About 3.5 mm across, a 15 mm close-up extracted from a 3D total-body photography capture, the subject is a male aged 63–67, the lesion is on the left lower leg, captured under cross-polarized illumination — 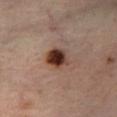Conclusion: On biopsy, histopathology showed a compound melanocytic nevus, classified as a benign lesion.